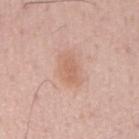workup — no biopsy performed (imaged during a skin exam)
image — ~15 mm tile from a whole-body skin photo
subject — male, about 55 years old
illumination — white-light illumination
site — the mid back